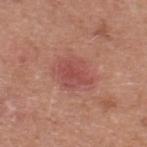The lesion was tiled from a total-body skin photograph and was not biopsied. Measured at roughly 4 mm in maximum diameter. A region of skin cropped from a whole-body photographic capture, roughly 15 mm wide. The lesion is on the upper back. A male patient about 50 years old.